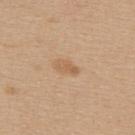Q: Was this lesion biopsied?
A: no biopsy performed (imaged during a skin exam)
Q: What are the patient's age and sex?
A: female, aged 38–42
Q: What is the imaging modality?
A: ~15 mm tile from a whole-body skin photo
Q: Lesion location?
A: the upper back